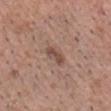Captured under white-light illumination.
Automated image analysis of the tile measured a footprint of about 3 mm² and a shape-asymmetry score of about 0.35 (0 = symmetric). And it measured an average lesion color of about L≈48 a*≈19 b*≈25 (CIELAB), roughly 10 lightness units darker than nearby skin, and a normalized lesion–skin contrast near 7.5. The analysis additionally found border irregularity of about 4 on a 0–10 scale, internal color variation of about 0 on a 0–10 scale, and peripheral color asymmetry of about 0. The analysis additionally found a lesion-detection confidence of about 100/100.
A male subject, roughly 40 years of age.
This image is a 15 mm lesion crop taken from a total-body photograph.
The lesion is on the head or neck.
The recorded lesion diameter is about 3 mm.